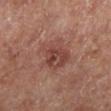| key | value |
|---|---|
| follow-up | catalogued during a skin exam; not biopsied |
| subject | male, aged 63–67 |
| location | the left lower leg |
| lesion size | ≈4.5 mm |
| image | total-body-photography crop, ~15 mm field of view |
| illumination | cross-polarized illumination |
| TBP lesion metrics | about 8 CIELAB-L* units darker than the surrounding skin; a peripheral color-asymmetry measure near 2 |